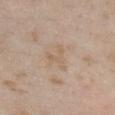Impression:
The lesion was photographed on a routine skin check and not biopsied; there is no pathology result.
Background:
A roughly 15 mm field-of-view crop from a total-body skin photograph. The lesion's longest dimension is about 3.5 mm. The lesion-visualizer software estimated a footprint of about 5 mm². And it measured a mean CIELAB color near L≈61 a*≈14 b*≈30, about 5 CIELAB-L* units darker than the surrounding skin, and a lesion-to-skin contrast of about 4.5 (normalized; higher = more distinct). And it measured a color-variation rating of about 1/10 and radial color variation of about 0. The lesion is located on the chest. Captured under white-light illumination. A female subject aged approximately 30.